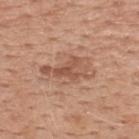Q: Is there a histopathology result?
A: imaged on a skin check; not biopsied
Q: What are the patient's age and sex?
A: male, in their 50s
Q: What did automated image analysis measure?
A: an eccentricity of roughly 0.9 and a shape-asymmetry score of about 0.55 (0 = symmetric)
Q: What kind of image is this?
A: ~15 mm crop, total-body skin-cancer survey
Q: Where on the body is the lesion?
A: the upper back
Q: What lighting was used for the tile?
A: white-light